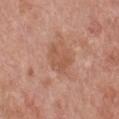Impression: This lesion was catalogued during total-body skin photography and was not selected for biopsy. Acquisition and patient details: An algorithmic analysis of the crop reported an area of roughly 7 mm² and two-axis asymmetry of about 0.3. It also reported a lesion color around L≈55 a*≈23 b*≈31 in CIELAB and a lesion-to-skin contrast of about 5.5 (normalized; higher = more distinct). It also reported a border-irregularity rating of about 3.5/10 and a peripheral color-asymmetry measure near 1. The tile uses white-light illumination. A 15 mm close-up tile from a total-body photography series done for melanoma screening. A female patient aged 58 to 62. The lesion is located on the chest.